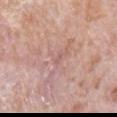workup: catalogued during a skin exam; not biopsied | automated lesion analysis: a lesion area of about 1.5 mm², an outline eccentricity of about 0.85 (0 = round, 1 = elongated), and a symmetry-axis asymmetry near 0.25; an average lesion color of about L≈59 a*≈22 b*≈23 (CIELAB), roughly 5 lightness units darker than nearby skin, and a normalized lesion–skin contrast near 3.5; border irregularity of about 2.5 on a 0–10 scale, a within-lesion color-variation index near 0/10, and a peripheral color-asymmetry measure near 0 | illumination: white-light | size: about 2 mm | image: ~15 mm crop, total-body skin-cancer survey | subject: male, aged 78 to 82 | anatomic site: the right upper arm.